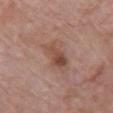Impression:
No biopsy was performed on this lesion — it was imaged during a full skin examination and was not determined to be concerning.
Context:
Located on the front of the torso. The patient is a male aged 78 to 82. Approximately 4.5 mm at its widest. A 15 mm close-up extracted from a 3D total-body photography capture. Automated image analysis of the tile measured a mean CIELAB color near L≈48 a*≈20 b*≈26, a lesion–skin lightness drop of about 10, and a normalized border contrast of about 7.5. It also reported a border-irregularity rating of about 3.5/10, a within-lesion color-variation index near 6/10, and radial color variation of about 2.5. It also reported a classifier nevus-likeness of about 40/100 and a lesion-detection confidence of about 100/100.